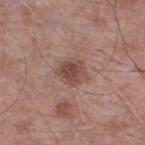| key | value |
|---|---|
| workup | no biopsy performed (imaged during a skin exam) |
| lesion diameter | about 3 mm |
| image | ~15 mm crop, total-body skin-cancer survey |
| automated metrics | an average lesion color of about L≈47 a*≈21 b*≈24 (CIELAB) and about 10 CIELAB-L* units darker than the surrounding skin; a border-irregularity index near 2/10, internal color variation of about 4 on a 0–10 scale, and radial color variation of about 1.5; a lesion-detection confidence of about 100/100 |
| illumination | white-light illumination |
| subject | male, aged approximately 55 |
| body site | the left lower leg |